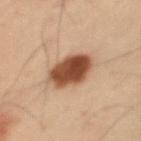| field | value |
|---|---|
| follow-up | no biopsy performed (imaged during a skin exam) |
| automated metrics | a lesion area of about 13 mm², a shape eccentricity near 0.5, and a shape-asymmetry score of about 0.15 (0 = symmetric); a mean CIELAB color near L≈38 a*≈19 b*≈27, about 16 CIELAB-L* units darker than the surrounding skin, and a lesion-to-skin contrast of about 13 (normalized; higher = more distinct); a border-irregularity rating of about 1.5/10, internal color variation of about 4.5 on a 0–10 scale, and a peripheral color-asymmetry measure near 1.5; an automated nevus-likeness rating near 100 out of 100 and a lesion-detection confidence of about 100/100 |
| illumination | cross-polarized |
| anatomic site | the left upper arm |
| image source | ~15 mm crop, total-body skin-cancer survey |
| lesion size | about 4.5 mm |
| patient | male, in their mid- to late 50s |